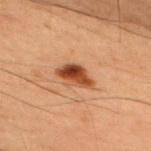| key | value |
|---|---|
| acquisition | total-body-photography crop, ~15 mm field of view |
| TBP lesion metrics | a lesion color around L≈36 a*≈22 b*≈30 in CIELAB, a lesion–skin lightness drop of about 13, and a normalized border contrast of about 11.5 |
| anatomic site | the right upper arm |
| lighting | cross-polarized illumination |
| diameter | ≈4 mm |
| patient | male, aged 58 to 62 |